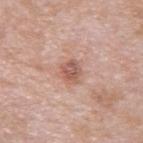• workup — no biopsy performed (imaged during a skin exam)
• TBP lesion metrics — a mean CIELAB color near L≈57 a*≈22 b*≈27; lesion-presence confidence of about 100/100
• lesion diameter — ~2.5 mm (longest diameter)
• image source — total-body-photography crop, ~15 mm field of view
• tile lighting — white-light illumination
• site — the upper back
• patient — male, aged 38 to 42